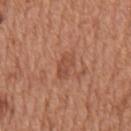{"biopsy_status": "not biopsied; imaged during a skin examination", "automated_metrics": {"cielab_L": 50, "cielab_a": 25, "cielab_b": 32, "vs_skin_darker_L": 7.0, "vs_skin_contrast_norm": 5.5}, "lesion_size": {"long_diameter_mm_approx": 3.5}, "lighting": "white-light", "site": "mid back", "patient": {"sex": "male", "age_approx": 65}, "image": {"source": "total-body photography crop", "field_of_view_mm": 15}}